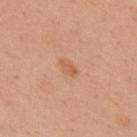Findings:
* notes — no biopsy performed (imaged during a skin exam)
* patient — female, aged approximately 30
* illumination — white-light illumination
* image — ~15 mm tile from a whole-body skin photo
* lesion size — ~2.5 mm (longest diameter)
* TBP lesion metrics — about 8 CIELAB-L* units darker than the surrounding skin and a normalized border contrast of about 5.5; a within-lesion color-variation index near 2/10 and radial color variation of about 0.5; a nevus-likeness score of about 10/100 and lesion-presence confidence of about 100/100
* location — the right upper arm